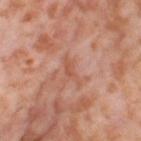Clinical impression:
Part of a total-body skin-imaging series; this lesion was reviewed on a skin check and was not flagged for biopsy.
Acquisition and patient details:
A 15 mm close-up extracted from a 3D total-body photography capture. The lesion's longest dimension is about 3.5 mm. Imaged with cross-polarized lighting. The lesion is located on the right thigh. The total-body-photography lesion software estimated an outline eccentricity of about 0.95 (0 = round, 1 = elongated) and two-axis asymmetry of about 0.45. It also reported about 7 CIELAB-L* units darker than the surrounding skin and a normalized lesion–skin contrast near 5.5. And it measured an automated nevus-likeness rating near 0 out of 100 and lesion-presence confidence of about 80/100. The subject is a female about 55 years old.